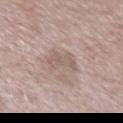notes = imaged on a skin check; not biopsied
diameter = about 3.5 mm
subject = male, aged 58 to 62
tile lighting = white-light
imaging modality = ~15 mm crop, total-body skin-cancer survey
image-analysis metrics = an area of roughly 5.5 mm², an eccentricity of roughly 0.8, and two-axis asymmetry of about 0.4; a lesion color around L≈58 a*≈15 b*≈22 in CIELAB and about 7 CIELAB-L* units darker than the surrounding skin; a classifier nevus-likeness of about 0/100 and a detector confidence of about 90 out of 100 that the crop contains a lesion
location = the right upper arm